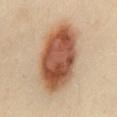{"biopsy_status": "not biopsied; imaged during a skin examination", "image": {"source": "total-body photography crop", "field_of_view_mm": 15}, "site": "mid back", "lighting": "cross-polarized", "patient": {"sex": "female", "age_approx": 40}}